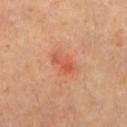| feature | finding |
|---|---|
| biopsy status | total-body-photography surveillance lesion; no biopsy |
| anatomic site | the leg |
| patient | female, aged 38 to 42 |
| image | 15 mm crop, total-body photography |
| illumination | cross-polarized illumination |